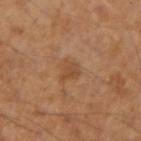Recorded during total-body skin imaging; not selected for excision or biopsy.
The patient is a male in their mid-40s.
A close-up tile cropped from a whole-body skin photograph, about 15 mm across.
The lesion is on the right forearm.
The tile uses cross-polarized illumination.